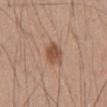{"biopsy_status": "not biopsied; imaged during a skin examination", "site": "abdomen", "lesion_size": {"long_diameter_mm_approx": 3.5}, "lighting": "white-light", "automated_metrics": {"area_mm2_approx": 6.0, "eccentricity": 0.7, "shape_asymmetry": 0.2, "cielab_L": 51, "cielab_a": 21, "cielab_b": 30, "vs_skin_darker_L": 11.0}, "patient": {"sex": "male", "age_approx": 55}, "image": {"source": "total-body photography crop", "field_of_view_mm": 15}}